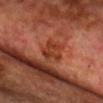Impression: The lesion was tiled from a total-body skin photograph and was not biopsied. Clinical summary: A 15 mm close-up tile from a total-body photography series done for melanoma screening. An algorithmic analysis of the crop reported a lesion area of about 5 mm² and an outline eccentricity of about 0.55 (0 = round, 1 = elongated). It also reported a mean CIELAB color near L≈38 a*≈29 b*≈35 and a normalized lesion–skin contrast near 6.5. The analysis additionally found border irregularity of about 8 on a 0–10 scale. The analysis additionally found an automated nevus-likeness rating near 0 out of 100 and a detector confidence of about 95 out of 100 that the crop contains a lesion. The lesion is on the chest. A male subject roughly 70 years of age.